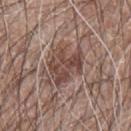Case summary:
- workup — imaged on a skin check; not biopsied
- size — about 5 mm
- patient — male, about 60 years old
- image — total-body-photography crop, ~15 mm field of view
- location — the arm